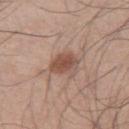<tbp_lesion>
  <biopsy_status>not biopsied; imaged during a skin examination</biopsy_status>
  <site>right thigh</site>
  <lesion_size>
    <long_diameter_mm_approx>3.5</long_diameter_mm_approx>
  </lesion_size>
  <patient>
    <sex>male</sex>
    <age_approx>45</age_approx>
  </patient>
  <automated_metrics>
    <area_mm2_approx>8.0</area_mm2_approx>
    <shape_asymmetry>0.25</shape_asymmetry>
    <nevus_likeness_0_100>85</nevus_likeness_0_100>
    <lesion_detection_confidence_0_100>100</lesion_detection_confidence_0_100>
  </automated_metrics>
  <image>
    <source>total-body photography crop</source>
    <field_of_view_mm>15</field_of_view_mm>
  </image>
  <lighting>white-light</lighting>
</tbp_lesion>